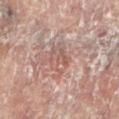Imaged during a routine full-body skin examination; the lesion was not biopsied and no histopathology is available. From the right leg. A female subject roughly 80 years of age. Longest diameter approximately 3 mm. The total-body-photography lesion software estimated an area of roughly 7 mm² and an eccentricity of roughly 0.35. The analysis additionally found border irregularity of about 2 on a 0–10 scale, a within-lesion color-variation index near 4.5/10, and peripheral color asymmetry of about 1.5. The analysis additionally found a classifier nevus-likeness of about 0/100 and lesion-presence confidence of about 60/100. Cropped from a whole-body photographic skin survey; the tile spans about 15 mm. Imaged with cross-polarized lighting.